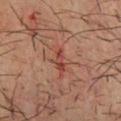Image and clinical context:
A close-up tile cropped from a whole-body skin photograph, about 15 mm across. On the front of the torso. The subject is a male aged approximately 35.